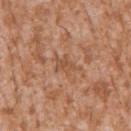Notes:
- follow-up — catalogued during a skin exam; not biopsied
- imaging modality — ~15 mm tile from a whole-body skin photo
- subject — male, about 45 years old
- site — the left upper arm